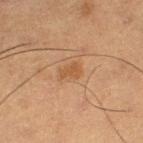Part of a total-body skin-imaging series; this lesion was reviewed on a skin check and was not flagged for biopsy. Automated tile analysis of the lesion measured an automated nevus-likeness rating near 15 out of 100. The subject is a male aged approximately 55. This is a cross-polarized tile. A 15 mm close-up tile from a total-body photography series done for melanoma screening. Located on the left thigh.